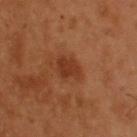Findings:
* image · total-body-photography crop, ~15 mm field of view
* body site · the upper back
* tile lighting · cross-polarized illumination
* lesion size · ~3.5 mm (longest diameter)
* subject · male, approximately 55 years of age